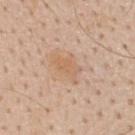| field | value |
|---|---|
| biopsy status | catalogued during a skin exam; not biopsied |
| image | ~15 mm tile from a whole-body skin photo |
| body site | the back |
| patient | male, aged approximately 60 |